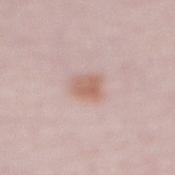Impression: Captured during whole-body skin photography for melanoma surveillance; the lesion was not biopsied. Image and clinical context: A region of skin cropped from a whole-body photographic capture, roughly 15 mm wide. From the abdomen. A male patient, aged 63–67. An algorithmic analysis of the crop reported a lesion area of about 6 mm², a shape eccentricity near 0.45, and a shape-asymmetry score of about 0.2 (0 = symmetric). The software also gave a border-irregularity index near 2/10 and radial color variation of about 1. It also reported an automated nevus-likeness rating near 85 out of 100 and a lesion-detection confidence of about 100/100. This is a white-light tile.